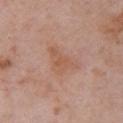Captured during whole-body skin photography for melanoma surveillance; the lesion was not biopsied.
Imaged with white-light lighting.
From the right upper arm.
A male patient, aged around 55.
Approximately 4.5 mm at its widest.
This image is a 15 mm lesion crop taken from a total-body photograph.